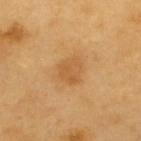Notes:
* biopsy status: imaged on a skin check; not biopsied
* size: about 3 mm
* imaging modality: ~15 mm crop, total-body skin-cancer survey
* patient: male, approximately 60 years of age
* anatomic site: the upper back
* lighting: cross-polarized